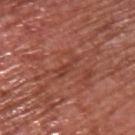biopsy status = no biopsy performed (imaged during a skin exam).